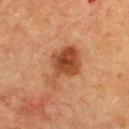Q: How was the tile lit?
A: cross-polarized illumination
Q: What are the patient's age and sex?
A: female, roughly 55 years of age
Q: What kind of image is this?
A: 15 mm crop, total-body photography
Q: Automated lesion metrics?
A: border irregularity of about 4.5 on a 0–10 scale and radial color variation of about 1.5; a nevus-likeness score of about 80/100 and a detector confidence of about 100 out of 100 that the crop contains a lesion
Q: Lesion location?
A: the upper back